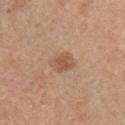biopsy status: catalogued during a skin exam; not biopsied | body site: the chest | image source: ~15 mm tile from a whole-body skin photo | TBP lesion metrics: a mean CIELAB color near L≈54 a*≈20 b*≈33, a lesion–skin lightness drop of about 9, and a normalized border contrast of about 6.5; border irregularity of about 2.5 on a 0–10 scale, internal color variation of about 2 on a 0–10 scale, and radial color variation of about 0.5; a nevus-likeness score of about 45/100 and a detector confidence of about 100 out of 100 that the crop contains a lesion | tile lighting: white-light | diameter: ≈3 mm | subject: male, aged 28 to 32.